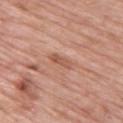Background: Imaged with white-light lighting. Approximately 3 mm at its widest. A female subject, approximately 65 years of age. The total-body-photography lesion software estimated a mean CIELAB color near L≈55 a*≈25 b*≈31, roughly 9 lightness units darker than nearby skin, and a normalized border contrast of about 6.5. The software also gave a border-irregularity rating of about 4/10, a color-variation rating of about 0/10, and peripheral color asymmetry of about 0. On the upper back. A lesion tile, about 15 mm wide, cut from a 3D total-body photograph.